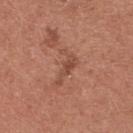Captured during whole-body skin photography for melanoma surveillance; the lesion was not biopsied. The patient is a female aged around 35. The recorded lesion diameter is about 4.5 mm. Located on the chest. A close-up tile cropped from a whole-body skin photograph, about 15 mm across.